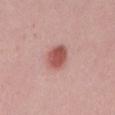| feature | finding |
|---|---|
| acquisition | total-body-photography crop, ~15 mm field of view |
| subject | female, aged approximately 15 |
| anatomic site | the upper back |